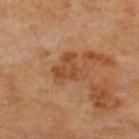{
  "biopsy_status": "not biopsied; imaged during a skin examination",
  "lesion_size": {
    "long_diameter_mm_approx": 4.0
  },
  "image": {
    "source": "total-body photography crop",
    "field_of_view_mm": 15
  },
  "lighting": "cross-polarized",
  "site": "back",
  "patient": {
    "sex": "male",
    "age_approx": 70
  }
}